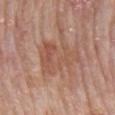  biopsy_status: not biopsied; imaged during a skin examination
  patient:
    sex: male
    age_approx: 75
  site: back
  lesion_size:
    long_diameter_mm_approx: 6.0
  image:
    source: total-body photography crop
    field_of_view_mm: 15
  automated_metrics:
    lesion_detection_confidence_0_100: 100